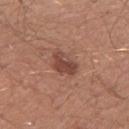Impression:
Imaged during a routine full-body skin examination; the lesion was not biopsied and no histopathology is available.
Background:
From the right upper arm. Cropped from a whole-body photographic skin survey; the tile spans about 15 mm. The patient is a male aged 28 to 32.